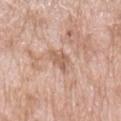Case summary:
– notes — catalogued during a skin exam; not biopsied
– patient — female, approximately 70 years of age
– lesion size — ~3 mm (longest diameter)
– automated metrics — a footprint of about 4 mm² and an eccentricity of roughly 0.7; a classifier nevus-likeness of about 0/100
– lighting — white-light
– anatomic site — the left upper arm
– image — ~15 mm tile from a whole-body skin photo